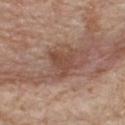Part of a total-body skin-imaging series; this lesion was reviewed on a skin check and was not flagged for biopsy.
The lesion's longest dimension is about 3 mm.
A lesion tile, about 15 mm wide, cut from a 3D total-body photograph.
A male patient, aged 78 to 82.
Automated tile analysis of the lesion measured a footprint of about 6 mm², a shape eccentricity near 0.5, and a shape-asymmetry score of about 0.55 (0 = symmetric). The analysis additionally found a border-irregularity rating of about 5.5/10, internal color variation of about 2 on a 0–10 scale, and peripheral color asymmetry of about 0.5.
The lesion is located on the upper back.
Imaged with white-light lighting.